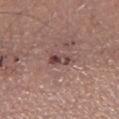notes = imaged on a skin check; not biopsied
subject = male, roughly 55 years of age
site = the right lower leg
acquisition = 15 mm crop, total-body photography